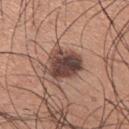<lesion>
<biopsy_status>not biopsied; imaged during a skin examination</biopsy_status>
<patient>
  <sex>male</sex>
  <age_approx>35</age_approx>
</patient>
<image>
  <source>total-body photography crop</source>
  <field_of_view_mm>15</field_of_view_mm>
</image>
<site>right upper arm</site>
</lesion>